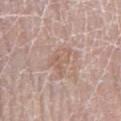* workup: no biopsy performed (imaged during a skin exam)
* acquisition: ~15 mm crop, total-body skin-cancer survey
* site: the left lower leg
* lighting: white-light
* automated metrics: a footprint of about 5 mm², a shape eccentricity near 0.8, and a symmetry-axis asymmetry near 0.6; a mean CIELAB color near L≈59 a*≈18 b*≈26 and a lesion–skin lightness drop of about 6; a border-irregularity rating of about 6.5/10 and peripheral color asymmetry of about 1
* subject: female, aged 58–62
* lesion diameter: about 3.5 mm